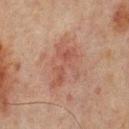No biopsy was performed on this lesion — it was imaged during a full skin examination and was not determined to be concerning.
The patient is a male aged 63 to 67.
This is a cross-polarized tile.
Automated image analysis of the tile measured a mean CIELAB color near L≈42 a*≈19 b*≈23, about 6 CIELAB-L* units darker than the surrounding skin, and a lesion-to-skin contrast of about 5.5 (normalized; higher = more distinct). The software also gave a classifier nevus-likeness of about 5/100 and a detector confidence of about 100 out of 100 that the crop contains a lesion.
A region of skin cropped from a whole-body photographic capture, roughly 15 mm wide.
Located on the back.